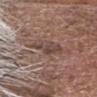Captured during whole-body skin photography for melanoma surveillance; the lesion was not biopsied.
A lesion tile, about 15 mm wide, cut from a 3D total-body photograph.
A male patient, aged approximately 75.
The lesion is located on the head or neck.
About 4 mm across.